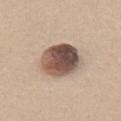Assessment:
Captured during whole-body skin photography for melanoma surveillance; the lesion was not biopsied.
Acquisition and patient details:
A female patient, aged 18–22. The lesion is located on the chest. A 15 mm close-up tile from a total-body photography series done for melanoma screening.